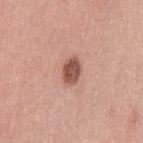Clinical impression:
Imaged during a routine full-body skin examination; the lesion was not biopsied and no histopathology is available.
Background:
A female patient about 50 years old. Located on the leg. Measured at roughly 3 mm in maximum diameter. Cropped from a total-body skin-imaging series; the visible field is about 15 mm. This is a white-light tile. An algorithmic analysis of the crop reported a footprint of about 5.5 mm² and a shape-asymmetry score of about 0.15 (0 = symmetric). The analysis additionally found a mean CIELAB color near L≈52 a*≈24 b*≈27 and a normalized lesion–skin contrast near 10. The analysis additionally found a border-irregularity rating of about 1.5/10, a within-lesion color-variation index near 3.5/10, and radial color variation of about 1. The software also gave a nevus-likeness score of about 90/100.